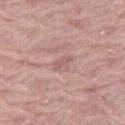Q: Was this lesion biopsied?
A: catalogued during a skin exam; not biopsied
Q: How was the tile lit?
A: white-light illumination
Q: What is the imaging modality?
A: total-body-photography crop, ~15 mm field of view
Q: What are the patient's age and sex?
A: female, aged around 70
Q: Lesion location?
A: the right thigh